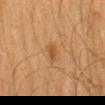{"biopsy_status": "not biopsied; imaged during a skin examination", "image": {"source": "total-body photography crop", "field_of_view_mm": 15}, "lesion_size": {"long_diameter_mm_approx": 2.5}, "patient": {"sex": "male", "age_approx": 65}, "site": "back", "lighting": "cross-polarized", "automated_metrics": {"shape_asymmetry": 0.2, "cielab_L": 43, "cielab_a": 18, "cielab_b": 33, "vs_skin_contrast_norm": 5.5}}